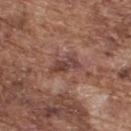This lesion was catalogued during total-body skin photography and was not selected for biopsy. The patient is a male aged around 75. A roughly 15 mm field-of-view crop from a total-body skin photograph. On the upper back.